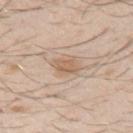| feature | finding |
|---|---|
| workup | no biopsy performed (imaged during a skin exam) |
| lesion diameter | about 2.5 mm |
| body site | the left upper arm |
| subject | male, aged 28 to 32 |
| automated metrics | a footprint of about 4.5 mm², an eccentricity of roughly 0.65, and two-axis asymmetry of about 0.3; an average lesion color of about L≈61 a*≈17 b*≈31 (CIELAB), a lesion–skin lightness drop of about 9, and a normalized lesion–skin contrast near 6.5 |
| image source | ~15 mm crop, total-body skin-cancer survey |